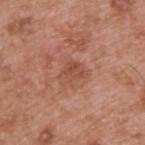Case summary:
- biopsy status: no biopsy performed (imaged during a skin exam)
- site: the upper back
- acquisition: total-body-photography crop, ~15 mm field of view
- patient: male, roughly 55 years of age